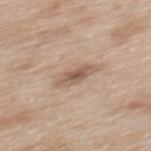Recorded during total-body skin imaging; not selected for excision or biopsy.
Captured under white-light illumination.
A lesion tile, about 15 mm wide, cut from a 3D total-body photograph.
The lesion is located on the back.
A female subject, about 40 years old.
Automated image analysis of the tile measured a footprint of about 6 mm² and a shape-asymmetry score of about 0.25 (0 = symmetric). The software also gave a lesion color around L≈57 a*≈16 b*≈28 in CIELAB, a lesion–skin lightness drop of about 11, and a normalized lesion–skin contrast near 7.